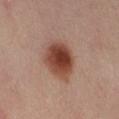Recorded during total-body skin imaging; not selected for excision or biopsy.
Automated image analysis of the tile measured a lesion area of about 15 mm². And it measured a mean CIELAB color near L≈43 a*≈23 b*≈28, about 15 CIELAB-L* units darker than the surrounding skin, and a lesion-to-skin contrast of about 11 (normalized; higher = more distinct). It also reported a border-irregularity index near 2/10 and a within-lesion color-variation index near 6.5/10.
The subject is a female aged around 40.
About 4.5 mm across.
The tile uses cross-polarized illumination.
Located on the right thigh.
Cropped from a whole-body photographic skin survey; the tile spans about 15 mm.